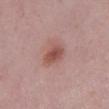| field | value |
|---|---|
| biopsy status | imaged on a skin check; not biopsied |
| illumination | white-light illumination |
| subject | female, aged 38–42 |
| lesion size | ~2.5 mm (longest diameter) |
| location | the right lower leg |
| imaging modality | total-body-photography crop, ~15 mm field of view |